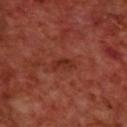| feature | finding |
|---|---|
| biopsy status | total-body-photography surveillance lesion; no biopsy |
| image | total-body-photography crop, ~15 mm field of view |
| patient | male, aged 68 to 72 |
| anatomic site | the upper back |
| lighting | cross-polarized illumination |